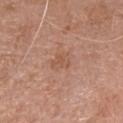Assessment:
Recorded during total-body skin imaging; not selected for excision or biopsy.
Context:
The tile uses white-light illumination. A female subject aged 73–77. From the head or neck. A 15 mm crop from a total-body photograph taken for skin-cancer surveillance. Measured at roughly 3 mm in maximum diameter.